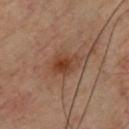No biopsy was performed on this lesion — it was imaged during a full skin examination and was not determined to be concerning. Approximately 3 mm at its widest. The lesion is on the front of the torso. Cropped from a total-body skin-imaging series; the visible field is about 15 mm. The tile uses cross-polarized illumination. The subject is a male aged around 55.